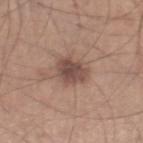Impression: Captured during whole-body skin photography for melanoma surveillance; the lesion was not biopsied. Image and clinical context: The lesion is on the right lower leg. Captured under white-light illumination. The total-body-photography lesion software estimated an area of roughly 9.5 mm², a shape eccentricity near 0.65, and a symmetry-axis asymmetry near 0.2. The software also gave a lesion color around L≈49 a*≈18 b*≈23 in CIELAB and a lesion–skin lightness drop of about 11. The software also gave a within-lesion color-variation index near 3.5/10 and radial color variation of about 1. The analysis additionally found a nevus-likeness score of about 70/100 and a detector confidence of about 100 out of 100 that the crop contains a lesion. A male subject, aged around 45. Cropped from a total-body skin-imaging series; the visible field is about 15 mm.